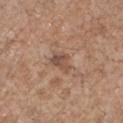Q: Was a biopsy performed?
A: no biopsy performed (imaged during a skin exam)
Q: What are the patient's age and sex?
A: male, about 70 years old
Q: What is the anatomic site?
A: the right upper arm
Q: Illumination type?
A: white-light illumination
Q: What kind of image is this?
A: ~15 mm crop, total-body skin-cancer survey
Q: Lesion size?
A: ≈2.5 mm